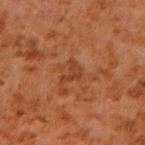{
  "site": "right upper arm",
  "patient": {
    "sex": "male",
    "age_approx": 60
  },
  "lighting": "cross-polarized",
  "image": {
    "source": "total-body photography crop",
    "field_of_view_mm": 15
  }
}